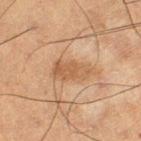Q: Was this lesion biopsied?
A: imaged on a skin check; not biopsied
Q: What is the imaging modality?
A: ~15 mm tile from a whole-body skin photo
Q: Automated lesion metrics?
A: an average lesion color of about L≈51 a*≈18 b*≈33 (CIELAB), about 8 CIELAB-L* units darker than the surrounding skin, and a normalized border contrast of about 6; an automated nevus-likeness rating near 5 out of 100 and a detector confidence of about 100 out of 100 that the crop contains a lesion
Q: What are the patient's age and sex?
A: male, about 55 years old
Q: How large is the lesion?
A: ≈4.5 mm
Q: Where on the body is the lesion?
A: the left thigh
Q: How was the tile lit?
A: cross-polarized